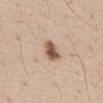follow-up: catalogued during a skin exam; not biopsied
image: ~15 mm crop, total-body skin-cancer survey
automated lesion analysis: a color-variation rating of about 4/10 and a peripheral color-asymmetry measure near 1; a classifier nevus-likeness of about 95/100 and a lesion-detection confidence of about 100/100
lesion size: ~3.5 mm (longest diameter)
location: the front of the torso
subject: male, aged approximately 40
lighting: white-light illumination